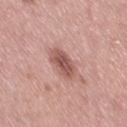<tbp_lesion>
  <biopsy_status>not biopsied; imaged during a skin examination</biopsy_status>
  <site>right thigh</site>
  <image>
    <source>total-body photography crop</source>
    <field_of_view_mm>15</field_of_view_mm>
  </image>
  <lesion_size>
    <long_diameter_mm_approx>4.5</long_diameter_mm_approx>
  </lesion_size>
  <patient>
    <sex>female</sex>
    <age_approx>40</age_approx>
  </patient>
  <lighting>white-light</lighting>
</tbp_lesion>